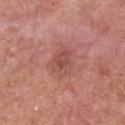Clinical impression:
The lesion was photographed on a routine skin check and not biopsied; there is no pathology result.
Context:
The tile uses white-light illumination. The patient is a male in their mid- to late 50s. Longest diameter approximately 4 mm. A close-up tile cropped from a whole-body skin photograph, about 15 mm across. From the chest.